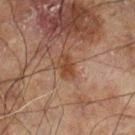Clinical impression:
The lesion was tiled from a total-body skin photograph and was not biopsied.
Background:
This image is a 15 mm lesion crop taken from a total-body photograph. A male patient, aged 58 to 62. From the left leg. Imaged with cross-polarized lighting. Automated image analysis of the tile measured an area of roughly 2.5 mm² and two-axis asymmetry of about 0.4. It also reported border irregularity of about 4.5 on a 0–10 scale, a within-lesion color-variation index near 0.5/10, and peripheral color asymmetry of about 0.